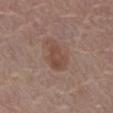Acquisition and patient details:
The tile uses white-light illumination. The subject is a male aged 78–82. The lesion is on the abdomen. The lesion's longest dimension is about 4.5 mm. Cropped from a total-body skin-imaging series; the visible field is about 15 mm.